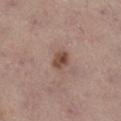{"biopsy_status": "not biopsied; imaged during a skin examination", "lesion_size": {"long_diameter_mm_approx": 2.5}, "lighting": "white-light", "automated_metrics": {"area_mm2_approx": 4.0, "eccentricity": 0.6, "shape_asymmetry": 0.2}, "image": {"source": "total-body photography crop", "field_of_view_mm": 15}, "patient": {"sex": "female", "age_approx": 65}, "site": "right lower leg"}